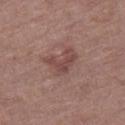Notes:
- workup: no biopsy performed (imaged during a skin exam)
- subject: male, approximately 70 years of age
- location: the right thigh
- size: ≈4 mm
- image source: ~15 mm tile from a whole-body skin photo